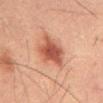Impression: Imaged during a routine full-body skin examination; the lesion was not biopsied and no histopathology is available.